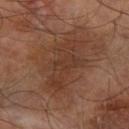Case summary:
• notes — catalogued during a skin exam; not biopsied
• image source — 15 mm crop, total-body photography
• lighting — cross-polarized illumination
• patient — male, aged 63 to 67
• location — the right forearm
• size — ~8 mm (longest diameter)